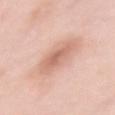No biopsy was performed on this lesion — it was imaged during a full skin examination and was not determined to be concerning. The subject is a female aged around 40. The tile uses white-light illumination. Located on the left upper arm. A 15 mm close-up extracted from a 3D total-body photography capture.